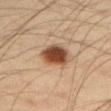notes: total-body-photography surveillance lesion; no biopsy
image source: ~15 mm tile from a whole-body skin photo
patient: male, approximately 35 years of age
location: the right upper arm
TBP lesion metrics: roughly 17 lightness units darker than nearby skin and a normalized lesion–skin contrast near 12.5; a border-irregularity index near 2/10, a within-lesion color-variation index near 5/10, and radial color variation of about 1.5; a classifier nevus-likeness of about 100/100 and a lesion-detection confidence of about 100/100
lighting: cross-polarized illumination